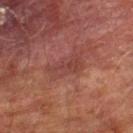Part of a total-body skin-imaging series; this lesion was reviewed on a skin check and was not flagged for biopsy. Cropped from a whole-body photographic skin survey; the tile spans about 15 mm. Automated tile analysis of the lesion measured an area of roughly 8 mm², an eccentricity of roughly 0.85, and a shape-asymmetry score of about 0.25 (0 = symmetric). It also reported a mean CIELAB color near L≈34 a*≈22 b*≈21, about 5 CIELAB-L* units darker than the surrounding skin, and a normalized border contrast of about 5. And it measured a nevus-likeness score of about 0/100 and a lesion-detection confidence of about 85/100. Captured under cross-polarized illumination. The lesion is located on the right lower leg. A male patient, aged approximately 75. Approximately 4.5 mm at its widest.